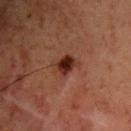biopsy status = catalogued during a skin exam; not biopsied
size = about 3 mm
subject = male, aged 58–62
acquisition = ~15 mm crop, total-body skin-cancer survey
site = the arm